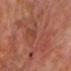Case summary:
• notes · total-body-photography surveillance lesion; no biopsy
• patient · male, aged around 60
• location · the front of the torso
• automated metrics · a classifier nevus-likeness of about 0/100 and lesion-presence confidence of about 90/100
• image · ~15 mm crop, total-body skin-cancer survey
• lesion size · ≈13 mm
• lighting · cross-polarized